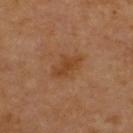Impression: The lesion was tiled from a total-body skin photograph and was not biopsied. Background: Approximately 4 mm at its widest. Imaged with cross-polarized lighting. A male patient roughly 70 years of age. The lesion-visualizer software estimated internal color variation of about 1.5 on a 0–10 scale and peripheral color asymmetry of about 0.5. A lesion tile, about 15 mm wide, cut from a 3D total-body photograph. Located on the upper back.